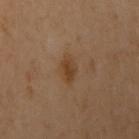biopsy status: imaged on a skin check; not biopsied | automated metrics: a footprint of about 4 mm² and a shape-asymmetry score of about 0.3 (0 = symmetric); a within-lesion color-variation index near 2.5/10 and a peripheral color-asymmetry measure near 1; an automated nevus-likeness rating near 75 out of 100 and a lesion-detection confidence of about 100/100 | patient: female, aged 58 to 62 | imaging modality: ~15 mm crop, total-body skin-cancer survey | lighting: cross-polarized illumination | size: about 2.5 mm | location: the upper back.